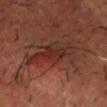Approximately 6 mm at its widest. A male subject aged approximately 50. A lesion tile, about 15 mm wide, cut from a 3D total-body photograph. The lesion is located on the head or neck. The total-body-photography lesion software estimated an area of roughly 14 mm², an outline eccentricity of about 0.85 (0 = round, 1 = elongated), and two-axis asymmetry of about 0.3. The software also gave an automated nevus-likeness rating near 5 out of 100 and a detector confidence of about 85 out of 100 that the crop contains a lesion.